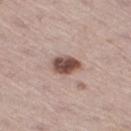The lesion was photographed on a routine skin check and not biopsied; there is no pathology result.
Imaged with white-light lighting.
The lesion is located on the right thigh.
A lesion tile, about 15 mm wide, cut from a 3D total-body photograph.
A male subject roughly 65 years of age.